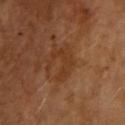A roughly 15 mm field-of-view crop from a total-body skin photograph. Approximately 4 mm at its widest. The lesion is located on the chest. The patient is a male in their mid-60s. Imaged with cross-polarized lighting. The total-body-photography lesion software estimated a lesion color around L≈36 a*≈22 b*≈34 in CIELAB and a lesion–skin lightness drop of about 6. The analysis additionally found a classifier nevus-likeness of about 0/100 and lesion-presence confidence of about 100/100.